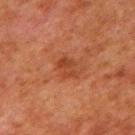Impression: Recorded during total-body skin imaging; not selected for excision or biopsy. Background: Longest diameter approximately 3 mm. Captured under cross-polarized illumination. A male subject, aged approximately 80. A 15 mm crop from a total-body photograph taken for skin-cancer surveillance. The lesion is on the mid back.